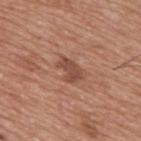Recorded during total-body skin imaging; not selected for excision or biopsy. The patient is a male aged 73–77. This image is a 15 mm lesion crop taken from a total-body photograph. From the upper back. About 3 mm across. The total-body-photography lesion software estimated a footprint of about 4.5 mm², an eccentricity of roughly 0.85, and a shape-asymmetry score of about 0.25 (0 = symmetric). And it measured a lesion–skin lightness drop of about 10 and a normalized border contrast of about 7.5. It also reported a within-lesion color-variation index near 2/10 and radial color variation of about 0.5. The analysis additionally found an automated nevus-likeness rating near 50 out of 100.